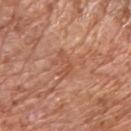| feature | finding |
|---|---|
| notes | catalogued during a skin exam; not biopsied |
| acquisition | ~15 mm tile from a whole-body skin photo |
| anatomic site | the arm |
| tile lighting | white-light illumination |
| diameter | ~3.5 mm (longest diameter) |
| patient | male, roughly 75 years of age |